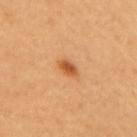<record>
<biopsy_status>not biopsied; imaged during a skin examination</biopsy_status>
<site>upper back</site>
<image>
  <source>total-body photography crop</source>
  <field_of_view_mm>15</field_of_view_mm>
</image>
<patient>
  <sex>female</sex>
  <age_approx>60</age_approx>
</patient>
</record>